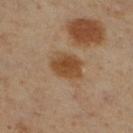Acquisition and patient details: This is a cross-polarized tile. Cropped from a whole-body photographic skin survey; the tile spans about 15 mm. Approximately 4 mm at its widest. From the leg. A male patient approximately 60 years of age. The total-body-photography lesion software estimated a lesion color around L≈42 a*≈17 b*≈32 in CIELAB, roughly 10 lightness units darker than nearby skin, and a normalized border contrast of about 9. It also reported border irregularity of about 2 on a 0–10 scale, a color-variation rating of about 2.5/10, and peripheral color asymmetry of about 1.